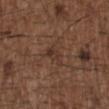image — 15 mm crop, total-body photography | lighting — white-light illumination | lesion diameter — ≈3 mm | location — the upper back | subject — male, roughly 50 years of age.